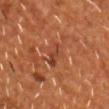Clinical summary:
Captured under cross-polarized illumination. A male patient, aged 53 to 57. The recorded lesion diameter is about 3.5 mm. An algorithmic analysis of the crop reported border irregularity of about 3.5 on a 0–10 scale, internal color variation of about 2.5 on a 0–10 scale, and a peripheral color-asymmetry measure near 0.5. The software also gave lesion-presence confidence of about 90/100. On the front of the torso. A 15 mm close-up extracted from a 3D total-body photography capture.